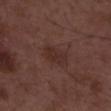Part of a total-body skin-imaging series; this lesion was reviewed on a skin check and was not flagged for biopsy.
Automated image analysis of the tile measured an eccentricity of roughly 0.85 and a symmetry-axis asymmetry near 0.45. The analysis additionally found a lesion color around L≈29 a*≈20 b*≈20 in CIELAB, a lesion–skin lightness drop of about 6, and a lesion-to-skin contrast of about 6 (normalized; higher = more distinct). And it measured a nevus-likeness score of about 10/100 and a lesion-detection confidence of about 100/100.
Measured at roughly 3.5 mm in maximum diameter.
The patient is a male roughly 50 years of age.
Captured under white-light illumination.
Cropped from a whole-body photographic skin survey; the tile spans about 15 mm.